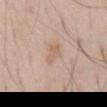follow-up: total-body-photography surveillance lesion; no biopsy
acquisition: ~15 mm tile from a whole-body skin photo
diameter: ≈3 mm
subject: male, aged 68 to 72
tile lighting: white-light
automated lesion analysis: an area of roughly 3.5 mm², an eccentricity of roughly 0.9, and a shape-asymmetry score of about 0.35 (0 = symmetric); border irregularity of about 3.5 on a 0–10 scale and a peripheral color-asymmetry measure near 0
site: the front of the torso